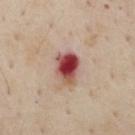{"biopsy_status": "not biopsied; imaged during a skin examination", "image": {"source": "total-body photography crop", "field_of_view_mm": 15}, "lighting": "cross-polarized", "patient": {"sex": "male", "age_approx": 55}, "site": "chest", "automated_metrics": {"cielab_L": 47, "cielab_a": 30, "cielab_b": 25, "vs_skin_contrast_norm": 13.0, "border_irregularity_0_10": 2.5, "color_variation_0_10": 10.0, "peripheral_color_asymmetry": 3.5, "nevus_likeness_0_100": 0, "lesion_detection_confidence_0_100": 100}, "lesion_size": {"long_diameter_mm_approx": 4.0}}